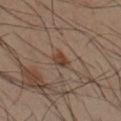follow-up: no biopsy performed (imaged during a skin exam) | lesion size: about 2.5 mm | tile lighting: cross-polarized | site: the left forearm | subject: male, approximately 40 years of age | image-analysis metrics: a lesion area of about 3.5 mm², an eccentricity of roughly 0.7, and two-axis asymmetry of about 0.3; border irregularity of about 3 on a 0–10 scale, a within-lesion color-variation index near 3/10, and peripheral color asymmetry of about 1 | image source: 15 mm crop, total-body photography.